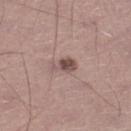Context:
On the left lower leg. A 15 mm crop from a total-body photograph taken for skin-cancer surveillance. The total-body-photography lesion software estimated a lesion color around L≈50 a*≈17 b*≈20 in CIELAB and roughly 12 lightness units darker than nearby skin. And it measured a border-irregularity index near 3.5/10 and internal color variation of about 3 on a 0–10 scale. It also reported a classifier nevus-likeness of about 70/100 and a lesion-detection confidence of about 100/100. A male subject, aged approximately 45. Measured at roughly 3 mm in maximum diameter.